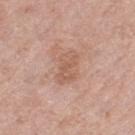  biopsy_status: not biopsied; imaged during a skin examination
  lesion_size:
    long_diameter_mm_approx: 4.0
  site: left thigh
  patient:
    sex: female
    age_approx: 70
  lighting: white-light
  image:
    source: total-body photography crop
    field_of_view_mm: 15